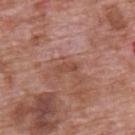Clinical impression:
Part of a total-body skin-imaging series; this lesion was reviewed on a skin check and was not flagged for biopsy.
Background:
The lesion is located on the upper back. A 15 mm close-up tile from a total-body photography series done for melanoma screening. The subject is a male aged around 70.